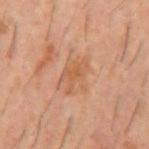Clinical impression: Part of a total-body skin-imaging series; this lesion was reviewed on a skin check and was not flagged for biopsy. Background: A male subject aged 58–62. Cropped from a total-body skin-imaging series; the visible field is about 15 mm. The lesion is on the back. This is a cross-polarized tile. Longest diameter approximately 4 mm.